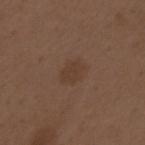Q: Was this lesion biopsied?
A: imaged on a skin check; not biopsied
Q: How large is the lesion?
A: ≈3 mm
Q: How was this image acquired?
A: ~15 mm tile from a whole-body skin photo
Q: Illumination type?
A: white-light
Q: Patient demographics?
A: male, aged approximately 70
Q: Lesion location?
A: the left upper arm